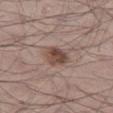The lesion was photographed on a routine skin check and not biopsied; there is no pathology result. A male subject aged 68 to 72. Measured at roughly 3 mm in maximum diameter. A region of skin cropped from a whole-body photographic capture, roughly 15 mm wide. On the leg. The lesion-visualizer software estimated a lesion area of about 6.5 mm², a shape eccentricity near 0.35, and two-axis asymmetry of about 0.2. And it measured a border-irregularity rating of about 2/10 and peripheral color asymmetry of about 2. It also reported a lesion-detection confidence of about 100/100. This is a white-light tile.